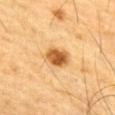No biopsy was performed on this lesion — it was imaged during a full skin examination and was not determined to be concerning.
The patient is a male aged around 85 or older.
A 15 mm close-up extracted from a 3D total-body photography capture.
The recorded lesion diameter is about 3 mm.
On the chest.